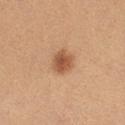Findings:
- follow-up — total-body-photography surveillance lesion; no biopsy
- image source — ~15 mm crop, total-body skin-cancer survey
- anatomic site — the chest
- tile lighting — white-light illumination
- TBP lesion metrics — a mean CIELAB color near L≈55 a*≈23 b*≈35, roughly 12 lightness units darker than nearby skin, and a normalized border contrast of about 8.5; a detector confidence of about 100 out of 100 that the crop contains a lesion
- subject — female, aged 23 to 27